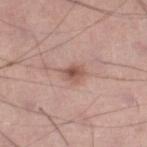Assessment: Imaged during a routine full-body skin examination; the lesion was not biopsied and no histopathology is available. Context: The lesion's longest dimension is about 3 mm. A male patient aged around 50. A lesion tile, about 15 mm wide, cut from a 3D total-body photograph. Automated image analysis of the tile measured a footprint of about 4.5 mm², a shape eccentricity near 0.65, and a shape-asymmetry score of about 0.25 (0 = symmetric). It also reported about 11 CIELAB-L* units darker than the surrounding skin and a normalized border contrast of about 7.5. Located on the right lower leg. Imaged with white-light lighting.